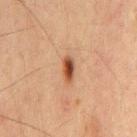A lesion tile, about 15 mm wide, cut from a 3D total-body photograph.
The tile uses cross-polarized illumination.
A male subject, roughly 60 years of age.